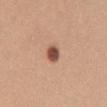workup: total-body-photography surveillance lesion; no biopsy | patient: female, in their 20s | size: about 2.5 mm | site: the front of the torso | illumination: white-light | image: 15 mm crop, total-body photography.